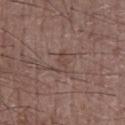A close-up tile cropped from a whole-body skin photograph, about 15 mm across. A male patient aged 58 to 62. The lesion-visualizer software estimated a shape eccentricity near 0.9 and a symmetry-axis asymmetry near 0.35. And it measured a mean CIELAB color near L≈44 a*≈16 b*≈22, roughly 6 lightness units darker than nearby skin, and a lesion-to-skin contrast of about 5 (normalized; higher = more distinct). It also reported a detector confidence of about 80 out of 100 that the crop contains a lesion. About 3 mm across. Located on the chest.